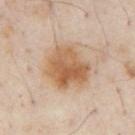{"biopsy_status": "not biopsied; imaged during a skin examination", "image": {"source": "total-body photography crop", "field_of_view_mm": 15}, "site": "chest", "patient": {"sex": "male", "age_approx": 55}, "lighting": "cross-polarized"}